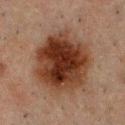Part of a total-body skin-imaging series; this lesion was reviewed on a skin check and was not flagged for biopsy.